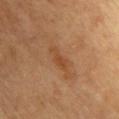notes=no biopsy performed (imaged during a skin exam) | lesion diameter=about 3.5 mm | site=the chest | lighting=cross-polarized illumination | patient=male, roughly 65 years of age | image source=~15 mm tile from a whole-body skin photo.